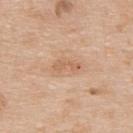Imaged during a routine full-body skin examination; the lesion was not biopsied and no histopathology is available.
A male patient in their mid- to late 60s.
This is a white-light tile.
The lesion is on the upper back.
A roughly 15 mm field-of-view crop from a total-body skin photograph.
Approximately 3 mm at its widest.